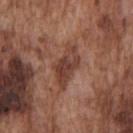Recorded during total-body skin imaging; not selected for excision or biopsy. The tile uses white-light illumination. Measured at roughly 5.5 mm in maximum diameter. A close-up tile cropped from a whole-body skin photograph, about 15 mm across. A male patient, in their mid- to late 70s. From the chest.